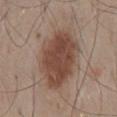  patient:
    sex: male
    age_approx: 55
  site: mid back
  image:
    source: total-body photography crop
    field_of_view_mm: 15